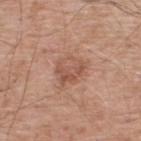notes = catalogued during a skin exam; not biopsied | location = the upper back | image = ~15 mm crop, total-body skin-cancer survey | patient = male, in their mid- to late 50s | lighting = white-light.